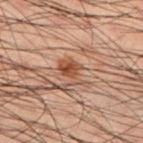The subject is a male about 50 years old. A 15 mm close-up tile from a total-body photography series done for melanoma screening. From the left lower leg.